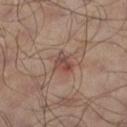Notes:
- biopsy status — no biopsy performed (imaged during a skin exam)
- illumination — cross-polarized
- lesion size — ~3 mm (longest diameter)
- body site — the leg
- patient — male, aged approximately 65
- acquisition — ~15 mm tile from a whole-body skin photo